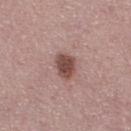Recorded during total-body skin imaging; not selected for excision or biopsy. A close-up tile cropped from a whole-body skin photograph, about 15 mm across. Longest diameter approximately 3 mm. Captured under white-light illumination. A male subject, aged 28–32. Automated image analysis of the tile measured a lesion color around L≈47 a*≈21 b*≈22 in CIELAB, a lesion–skin lightness drop of about 14, and a normalized border contrast of about 10. The analysis additionally found border irregularity of about 1.5 on a 0–10 scale, a within-lesion color-variation index near 4/10, and a peripheral color-asymmetry measure near 1.